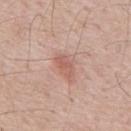Recorded during total-body skin imaging; not selected for excision or biopsy. The tile uses white-light illumination. A male patient, about 75 years old. The lesion is on the mid back. Measured at roughly 3 mm in maximum diameter. Cropped from a whole-body photographic skin survey; the tile spans about 15 mm.